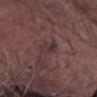{"image": {"source": "total-body photography crop", "field_of_view_mm": 15}, "patient": {"sex": "female", "age_approx": 35}, "lesion_size": {"long_diameter_mm_approx": 3.0}, "lighting": "white-light", "site": "front of the torso"}